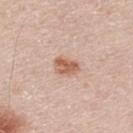Clinical impression: Recorded during total-body skin imaging; not selected for excision or biopsy. Acquisition and patient details: The lesion is on the upper back. A male subject aged around 40. A roughly 15 mm field-of-view crop from a total-body skin photograph.